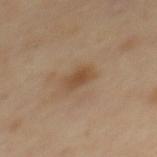| field | value |
|---|---|
| notes | catalogued during a skin exam; not biopsied |
| anatomic site | the upper back |
| imaging modality | 15 mm crop, total-body photography |
| lighting | cross-polarized illumination |
| subject | female, roughly 40 years of age |
| diameter | ≈3 mm |